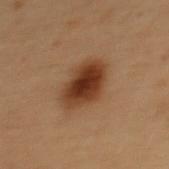Assessment:
This lesion was catalogued during total-body skin photography and was not selected for biopsy.
Context:
The lesion-visualizer software estimated an average lesion color of about L≈32 a*≈19 b*≈28 (CIELAB), a lesion–skin lightness drop of about 13, and a lesion-to-skin contrast of about 11.5 (normalized; higher = more distinct). It also reported an automated nevus-likeness rating near 100 out of 100 and a detector confidence of about 100 out of 100 that the crop contains a lesion. This is a cross-polarized tile. A 15 mm crop from a total-body photograph taken for skin-cancer surveillance. The lesion is located on the back. A female subject aged around 50. About 4.5 mm across.